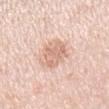workup: no biopsy performed (imaged during a skin exam)
lesion size: about 4.5 mm
subject: male, aged 58–62
site: the right upper arm
image: ~15 mm crop, total-body skin-cancer survey
image-analysis metrics: a lesion area of about 9.5 mm², an eccentricity of roughly 0.8, and a symmetry-axis asymmetry near 0.2; a detector confidence of about 100 out of 100 that the crop contains a lesion